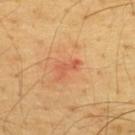Recorded during total-body skin imaging; not selected for excision or biopsy. An algorithmic analysis of the crop reported a lesion area of about 3 mm², a shape eccentricity near 0.9, and two-axis asymmetry of about 0.55. The analysis additionally found a lesion color around L≈59 a*≈31 b*≈40 in CIELAB, roughly 8 lightness units darker than nearby skin, and a lesion-to-skin contrast of about 5.5 (normalized; higher = more distinct). It also reported a border-irregularity rating of about 6.5/10 and peripheral color asymmetry of about 0. A male subject in their mid- to late 60s. A lesion tile, about 15 mm wide, cut from a 3D total-body photograph. The lesion is on the upper back. Longest diameter approximately 3 mm.